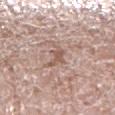notes = catalogued during a skin exam; not biopsied
subject = male, aged 58 to 62
image-analysis metrics = a mean CIELAB color near L≈56 a*≈18 b*≈25, roughly 9 lightness units darker than nearby skin, and a normalized lesion–skin contrast near 6.5; an automated nevus-likeness rating near 0 out of 100 and a lesion-detection confidence of about 60/100
image source = ~15 mm tile from a whole-body skin photo
body site = the right lower leg
size = ≈3.5 mm